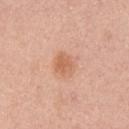follow-up = imaged on a skin check; not biopsied